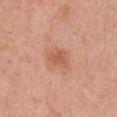workup: no biopsy performed (imaged during a skin exam)
acquisition: ~15 mm tile from a whole-body skin photo
automated metrics: a color-variation rating of about 1.5/10; a nevus-likeness score of about 45/100 and lesion-presence confidence of about 100/100
size: ≈3 mm
location: the chest
subject: female, aged 33 to 37